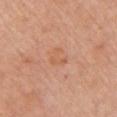Q: Was this lesion biopsied?
A: catalogued during a skin exam; not biopsied
Q: Patient demographics?
A: female, roughly 65 years of age
Q: How was the tile lit?
A: white-light
Q: Lesion size?
A: ≈2.5 mm
Q: How was this image acquired?
A: total-body-photography crop, ~15 mm field of view
Q: What did automated image analysis measure?
A: an eccentricity of roughly 0.6 and a shape-asymmetry score of about 0.55 (0 = symmetric); about 5 CIELAB-L* units darker than the surrounding skin
Q: Lesion location?
A: the chest